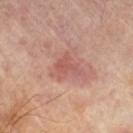No biopsy was performed on this lesion — it was imaged during a full skin examination and was not determined to be concerning. Measured at roughly 2.5 mm in maximum diameter. A male subject, aged around 70. Located on the right thigh. A 15 mm close-up tile from a total-body photography series done for melanoma screening.